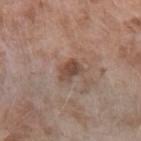  biopsy_status: not biopsied; imaged during a skin examination
  site: arm
  lesion_size:
    long_diameter_mm_approx: 3.0
  patient:
    sex: female
    age_approx: 75
  image:
    source: total-body photography crop
    field_of_view_mm: 15
  lighting: white-light